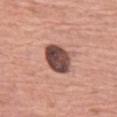Q: What kind of image is this?
A: total-body-photography crop, ~15 mm field of view
Q: What are the patient's age and sex?
A: female, aged approximately 65
Q: Illumination type?
A: white-light illumination
Q: Lesion size?
A: ~4.5 mm (longest diameter)
Q: Where on the body is the lesion?
A: the left thigh